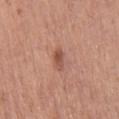Notes:
• biopsy status — no biopsy performed (imaged during a skin exam)
• anatomic site — the right upper arm
• image-analysis metrics — an outline eccentricity of about 0.85 (0 = round, 1 = elongated) and two-axis asymmetry of about 0.25; roughly 10 lightness units darker than nearby skin; lesion-presence confidence of about 100/100
• lesion diameter — ~2.5 mm (longest diameter)
• lighting — white-light illumination
• subject — male, aged around 55
• acquisition — ~15 mm crop, total-body skin-cancer survey